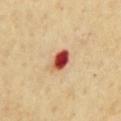biopsy status: total-body-photography surveillance lesion; no biopsy | lighting: cross-polarized | anatomic site: the chest | subject: male, approximately 65 years of age | lesion diameter: ≈3 mm | acquisition: ~15 mm crop, total-body skin-cancer survey.